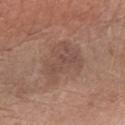Part of a total-body skin-imaging series; this lesion was reviewed on a skin check and was not flagged for biopsy. Measured at roughly 6 mm in maximum diameter. A male patient aged around 70. A 15 mm close-up extracted from a 3D total-body photography capture. The tile uses white-light illumination. Automated image analysis of the tile measured an average lesion color of about L≈49 a*≈18 b*≈25 (CIELAB) and a lesion–skin lightness drop of about 7. And it measured a border-irregularity rating of about 4/10, internal color variation of about 3 on a 0–10 scale, and radial color variation of about 1. The software also gave a classifier nevus-likeness of about 0/100 and a detector confidence of about 100 out of 100 that the crop contains a lesion. Located on the left forearm.